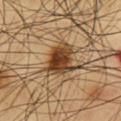image = 15 mm crop, total-body photography | diameter = ≈4.5 mm | lighting = cross-polarized | location = the chest | patient = male, about 60 years old.